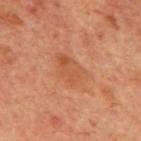Q: Was a biopsy performed?
A: imaged on a skin check; not biopsied
Q: What are the patient's age and sex?
A: male, aged 68 to 72
Q: What is the anatomic site?
A: the mid back
Q: What kind of image is this?
A: ~15 mm crop, total-body skin-cancer survey